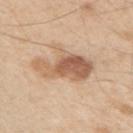| feature | finding |
|---|---|
| workup | no biopsy performed (imaged during a skin exam) |
| site | the right upper arm |
| subject | male, aged 48 to 52 |
| tile lighting | white-light |
| lesion size | ≈6.5 mm |
| acquisition | ~15 mm crop, total-body skin-cancer survey |